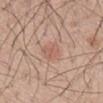Background:
A 15 mm close-up tile from a total-body photography series done for melanoma screening. A male subject aged approximately 50. The lesion is located on the back. Imaged with white-light lighting.